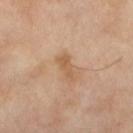Impression:
The lesion was tiled from a total-body skin photograph and was not biopsied.
Image and clinical context:
The total-body-photography lesion software estimated a footprint of about 4 mm², a shape eccentricity near 0.9, and a shape-asymmetry score of about 0.3 (0 = symmetric). The software also gave an automated nevus-likeness rating near 0 out of 100 and a detector confidence of about 100 out of 100 that the crop contains a lesion. About 3.5 mm across. Located on the leg. This is a cross-polarized tile. A 15 mm crop from a total-body photograph taken for skin-cancer surveillance. A female patient aged approximately 70.